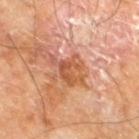biopsy status = total-body-photography surveillance lesion; no biopsy
image source = total-body-photography crop, ~15 mm field of view
tile lighting = cross-polarized illumination
anatomic site = the leg
TBP lesion metrics = an area of roughly 8.5 mm² and an eccentricity of roughly 0.65; a detector confidence of about 100 out of 100 that the crop contains a lesion
size = ≈4.5 mm
subject = male, approximately 60 years of age